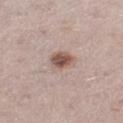follow-up: catalogued during a skin exam; not biopsied | acquisition: total-body-photography crop, ~15 mm field of view | lesion size: ~3.5 mm (longest diameter) | tile lighting: white-light illumination | anatomic site: the left thigh | subject: female, in their 30s | image-analysis metrics: a footprint of about 8 mm² and an outline eccentricity of about 0.45 (0 = round, 1 = elongated); an average lesion color of about L≈56 a*≈17 b*≈23 (CIELAB), roughly 11 lightness units darker than nearby skin, and a lesion-to-skin contrast of about 7.5 (normalized; higher = more distinct); a within-lesion color-variation index near 5.5/10 and peripheral color asymmetry of about 1.5; an automated nevus-likeness rating near 95 out of 100 and a detector confidence of about 100 out of 100 that the crop contains a lesion.